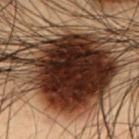follow-up: no biopsy performed (imaged during a skin exam)
site: the abdomen
patient: male, in their mid-50s
image source: ~15 mm crop, total-body skin-cancer survey